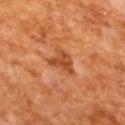- workup: no biopsy performed (imaged during a skin exam)
- site: the mid back
- diameter: ~3.5 mm (longest diameter)
- subject: male, aged around 65
- tile lighting: cross-polarized illumination
- imaging modality: ~15 mm crop, total-body skin-cancer survey